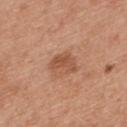Clinical impression:
Imaged during a routine full-body skin examination; the lesion was not biopsied and no histopathology is available.
Context:
A lesion tile, about 15 mm wide, cut from a 3D total-body photograph. The lesion-visualizer software estimated a lesion area of about 6.5 mm², an eccentricity of roughly 0.75, and two-axis asymmetry of about 0.3. And it measured a normalized lesion–skin contrast near 6.5. The software also gave a classifier nevus-likeness of about 10/100 and a lesion-detection confidence of about 100/100. This is a white-light tile. The recorded lesion diameter is about 3.5 mm. A male patient aged around 30. On the upper back.